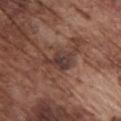{
  "biopsy_status": "not biopsied; imaged during a skin examination",
  "lesion_size": {
    "long_diameter_mm_approx": 3.5
  },
  "site": "chest",
  "lighting": "white-light",
  "patient": {
    "sex": "male",
    "age_approx": 75
  },
  "image": {
    "source": "total-body photography crop",
    "field_of_view_mm": 15
  }
}